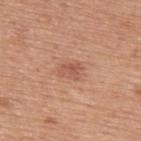Findings:
- biopsy status — catalogued during a skin exam; not biopsied
- subject — male, aged 73–77
- acquisition — ~15 mm tile from a whole-body skin photo
- site — the upper back
- illumination — white-light
- TBP lesion metrics — about 8 CIELAB-L* units darker than the surrounding skin and a lesion-to-skin contrast of about 5.5 (normalized; higher = more distinct); a border-irregularity rating of about 3/10, a within-lesion color-variation index near 3/10, and radial color variation of about 1; a nevus-likeness score of about 40/100 and lesion-presence confidence of about 100/100